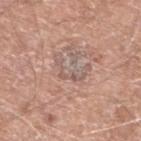Q: Was this lesion biopsied?
A: no biopsy performed (imaged during a skin exam)
Q: How was this image acquired?
A: total-body-photography crop, ~15 mm field of view
Q: Lesion location?
A: the left lower leg
Q: How large is the lesion?
A: about 4.5 mm
Q: What are the patient's age and sex?
A: male, about 80 years old
Q: How was the tile lit?
A: white-light illumination